The lesion is located on the mid back. Longest diameter approximately 4 mm. Captured under cross-polarized illumination. Cropped from a total-body skin-imaging series; the visible field is about 15 mm. A male patient, roughly 35 years of age — 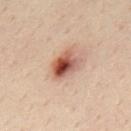On excision, pathology confirmed an atypical melanocytic neoplasm (borderline).